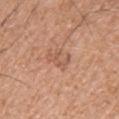Part of a total-body skin-imaging series; this lesion was reviewed on a skin check and was not flagged for biopsy. Cropped from a whole-body photographic skin survey; the tile spans about 15 mm. The patient is a male aged approximately 55. The lesion is on the upper back.